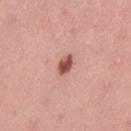Recorded during total-body skin imaging; not selected for excision or biopsy. The tile uses white-light illumination. A female subject, aged 33–37. Automated tile analysis of the lesion measured an area of roughly 3.5 mm², an eccentricity of roughly 0.8, and two-axis asymmetry of about 0.25. The analysis additionally found a within-lesion color-variation index near 3.5/10. The lesion's longest dimension is about 2.5 mm. Located on the left thigh. A 15 mm close-up extracted from a 3D total-body photography capture.